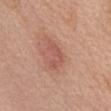A 15 mm close-up extracted from a 3D total-body photography capture.
Located on the front of the torso.
A female subject, about 60 years old.